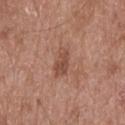Q: Is there a histopathology result?
A: total-body-photography surveillance lesion; no biopsy
Q: What are the patient's age and sex?
A: male, roughly 50 years of age
Q: What kind of image is this?
A: ~15 mm crop, total-body skin-cancer survey
Q: What is the lesion's diameter?
A: ~3.5 mm (longest diameter)
Q: What is the anatomic site?
A: the mid back
Q: Automated lesion metrics?
A: border irregularity of about 2.5 on a 0–10 scale, internal color variation of about 2 on a 0–10 scale, and peripheral color asymmetry of about 0.5; an automated nevus-likeness rating near 25 out of 100 and a detector confidence of about 100 out of 100 that the crop contains a lesion
Q: Illumination type?
A: white-light illumination